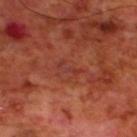Clinical summary: This is a cross-polarized tile. The recorded lesion diameter is about 3 mm. A 15 mm close-up tile from a total-body photography series done for melanoma screening. The subject is a male aged 68–72. The lesion is located on the upper back.